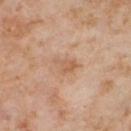Clinical impression: This lesion was catalogued during total-body skin photography and was not selected for biopsy. Background: A 15 mm crop from a total-body photograph taken for skin-cancer surveillance. A female patient aged 53 to 57. Captured under cross-polarized illumination. An algorithmic analysis of the crop reported an eccentricity of roughly 0.8 and a shape-asymmetry score of about 0.45 (0 = symmetric). The analysis additionally found a classifier nevus-likeness of about 0/100 and lesion-presence confidence of about 100/100. The lesion is located on the right thigh. The recorded lesion diameter is about 3 mm.